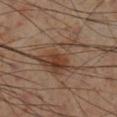The lesion was tiled from a total-body skin photograph and was not biopsied.
Measured at roughly 7 mm in maximum diameter.
Automated image analysis of the tile measured a lesion area of about 17 mm², an outline eccentricity of about 0.65 (0 = round, 1 = elongated), and a shape-asymmetry score of about 0.5 (0 = symmetric). And it measured an average lesion color of about L≈41 a*≈17 b*≈28 (CIELAB), roughly 9 lightness units darker than nearby skin, and a normalized lesion–skin contrast near 7.5. The software also gave a classifier nevus-likeness of about 65/100.
A male patient, roughly 55 years of age.
The tile uses cross-polarized illumination.
A 15 mm crop from a total-body photograph taken for skin-cancer surveillance.
On the leg.